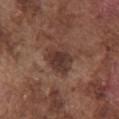Acquisition and patient details: The lesion is on the chest. A male subject, aged around 75. Cropped from a whole-body photographic skin survey; the tile spans about 15 mm.